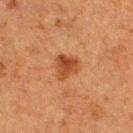Q: Is there a histopathology result?
A: no biopsy performed (imaged during a skin exam)
Q: Who is the patient?
A: male, aged 48 to 52
Q: Where on the body is the lesion?
A: the upper back
Q: How was this image acquired?
A: ~15 mm crop, total-body skin-cancer survey